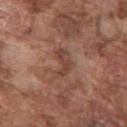– biopsy status — imaged on a skin check; not biopsied
– acquisition — ~15 mm tile from a whole-body skin photo
– patient — male, in their mid-70s
– size — about 4 mm
– illumination — white-light illumination
– body site — the right upper arm